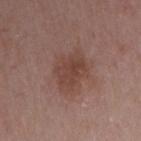Clinical impression:
Recorded during total-body skin imaging; not selected for excision or biopsy.
Image and clinical context:
On the right upper arm. Cropped from a total-body skin-imaging series; the visible field is about 15 mm. Captured under white-light illumination. About 4.5 mm across. A female subject, approximately 45 years of age.